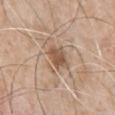The lesion was tiled from a total-body skin photograph and was not biopsied. An algorithmic analysis of the crop reported an area of roughly 5 mm², a shape eccentricity near 0.75, and a symmetry-axis asymmetry near 0.3. And it measured an average lesion color of about L≈53 a*≈18 b*≈30 (CIELAB), about 11 CIELAB-L* units darker than the surrounding skin, and a normalized border contrast of about 8. The subject is a male approximately 80 years of age. Approximately 3.5 mm at its widest. The lesion is located on the chest. Imaged with white-light lighting. A region of skin cropped from a whole-body photographic capture, roughly 15 mm wide.